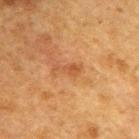biopsy_status: not biopsied; imaged during a skin examination
image:
  source: total-body photography crop
  field_of_view_mm: 15
patient:
  sex: male
  age_approx: 50
site: upper back